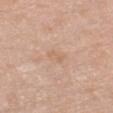notes — catalogued during a skin exam; not biopsied
anatomic site — the chest
subject — female, roughly 70 years of age
image-analysis metrics — a footprint of about 3.5 mm² and a shape eccentricity near 0.85; a mean CIELAB color near L≈64 a*≈18 b*≈32, roughly 5 lightness units darker than nearby skin, and a lesion-to-skin contrast of about 4.5 (normalized; higher = more distinct); a border-irregularity index near 2.5/10 and a within-lesion color-variation index near 1/10; a nevus-likeness score of about 0/100 and a lesion-detection confidence of about 100/100
tile lighting — white-light illumination
imaging modality — 15 mm crop, total-body photography